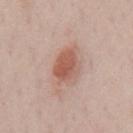Notes:
– biopsy status · imaged on a skin check; not biopsied
– automated metrics · a border-irregularity rating of about 2/10, a color-variation rating of about 4/10, and radial color variation of about 1
– lighting · white-light illumination
– site · the chest
– image · ~15 mm tile from a whole-body skin photo
– patient · male, aged approximately 50